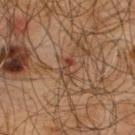  biopsy_status: not biopsied; imaged during a skin examination
  image:
    source: total-body photography crop
    field_of_view_mm: 15
  automated_metrics:
    border_irregularity_0_10: 5.5
    color_variation_0_10: 0.0
    peripheral_color_asymmetry: 0.0
  site: upper back
  patient:
    sex: male
    age_approx: 65
  lesion_size:
    long_diameter_mm_approx: 2.5
  lighting: cross-polarized